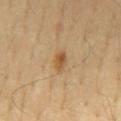  biopsy_status: not biopsied; imaged during a skin examination
  automated_metrics:
    area_mm2_approx: 3.0
    eccentricity: 0.8
    shape_asymmetry: 0.25
    cielab_L: 54
    cielab_a: 19
    cielab_b: 39
    vs_skin_darker_L: 10.0
    vs_skin_contrast_norm: 8.0
    border_irregularity_0_10: 2.5
    peripheral_color_asymmetry: 1.0
    nevus_likeness_0_100: 80
    lesion_detection_confidence_0_100: 100
  image:
    source: total-body photography crop
    field_of_view_mm: 15
  site: back
  patient:
    sex: male
    age_approx: 55